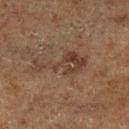The lesion's longest dimension is about 6.5 mm.
Automated tile analysis of the lesion measured a lesion color around L≈31 a*≈14 b*≈22 in CIELAB, a lesion–skin lightness drop of about 6, and a normalized lesion–skin contrast near 6.5. It also reported a border-irregularity rating of about 9.5/10 and peripheral color asymmetry of about 1.5. The software also gave an automated nevus-likeness rating near 0 out of 100 and a lesion-detection confidence of about 95/100.
The subject is a male aged 73 to 77.
A 15 mm close-up extracted from a 3D total-body photography capture.
The lesion is located on the left lower leg.
Captured under cross-polarized illumination.